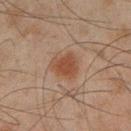Assessment:
Recorded during total-body skin imaging; not selected for excision or biopsy.
Image and clinical context:
From the left lower leg. About 3.5 mm across. Captured under cross-polarized illumination. A male patient in their mid-40s. A close-up tile cropped from a whole-body skin photograph, about 15 mm across. Automated image analysis of the tile measured about 8 CIELAB-L* units darker than the surrounding skin and a normalized lesion–skin contrast near 7.5.